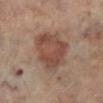From the left lower leg.
Captured under cross-polarized illumination.
A close-up tile cropped from a whole-body skin photograph, about 15 mm across.
About 6 mm across.
The subject is a female in their 60s.